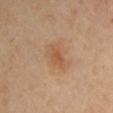Q: Was a biopsy performed?
A: catalogued during a skin exam; not biopsied
Q: Lesion location?
A: the arm
Q: What is the lesion's diameter?
A: ≈3.5 mm
Q: Who is the patient?
A: male, aged approximately 40
Q: What lighting was used for the tile?
A: cross-polarized illumination
Q: Automated lesion metrics?
A: a lesion area of about 5 mm², an eccentricity of roughly 0.85, and a shape-asymmetry score of about 0.25 (0 = symmetric); a lesion color around L≈54 a*≈20 b*≈35 in CIELAB, a lesion–skin lightness drop of about 7, and a normalized lesion–skin contrast near 6; a classifier nevus-likeness of about 30/100 and a detector confidence of about 100 out of 100 that the crop contains a lesion
Q: How was this image acquired?
A: total-body-photography crop, ~15 mm field of view